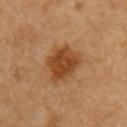The lesion was photographed on a routine skin check and not biopsied; there is no pathology result. From the arm. A close-up tile cropped from a whole-body skin photograph, about 15 mm across. This is a cross-polarized tile. About 4.5 mm across. A female patient aged around 70.